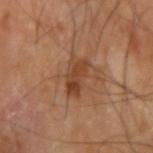Assessment: Part of a total-body skin-imaging series; this lesion was reviewed on a skin check and was not flagged for biopsy. Image and clinical context: A male subject, aged approximately 65. This image is a 15 mm lesion crop taken from a total-body photograph. The lesion is on the right upper arm.